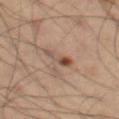Clinical impression: The lesion was photographed on a routine skin check and not biopsied; there is no pathology result. Image and clinical context: The lesion is on the left thigh. Imaged with cross-polarized lighting. The patient is a male aged 53 to 57. A region of skin cropped from a whole-body photographic capture, roughly 15 mm wide. An algorithmic analysis of the crop reported a classifier nevus-likeness of about 80/100 and a detector confidence of about 100 out of 100 that the crop contains a lesion.